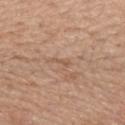Assessment: Imaged during a routine full-body skin examination; the lesion was not biopsied and no histopathology is available. Acquisition and patient details: From the upper back. Automated image analysis of the tile measured a classifier nevus-likeness of about 0/100 and a detector confidence of about 50 out of 100 that the crop contains a lesion. Longest diameter approximately 2.5 mm. A female patient aged approximately 60. Captured under white-light illumination. A roughly 15 mm field-of-view crop from a total-body skin photograph.